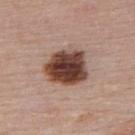• follow-up · imaged on a skin check; not biopsied
• illumination · white-light
• subject · female, about 50 years old
• location · the upper back
• image source · ~15 mm tile from a whole-body skin photo